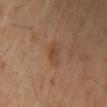From the right lower leg. A 15 mm close-up tile from a total-body photography series done for melanoma screening. A female patient aged 68 to 72.